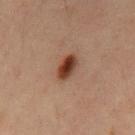<lesion>
<biopsy_status>not biopsied; imaged during a skin examination</biopsy_status>
<site>chest</site>
<image>
  <source>total-body photography crop</source>
  <field_of_view_mm>15</field_of_view_mm>
</image>
<lighting>cross-polarized</lighting>
<lesion_size>
  <long_diameter_mm_approx>3.5</long_diameter_mm_approx>
</lesion_size>
<patient>
  <sex>male</sex>
  <age_approx>60</age_approx>
</patient>
</lesion>